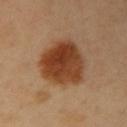A male subject, aged around 40. The lesion's longest dimension is about 6.5 mm. The tile uses cross-polarized illumination. A region of skin cropped from a whole-body photographic capture, roughly 15 mm wide. From the right upper arm. The total-body-photography lesion software estimated a footprint of about 25 mm², an outline eccentricity of about 0.55 (0 = round, 1 = elongated), and a shape-asymmetry score of about 0.2 (0 = symmetric). The software also gave a border-irregularity index near 2/10, a color-variation rating of about 6/10, and a peripheral color-asymmetry measure near 2.